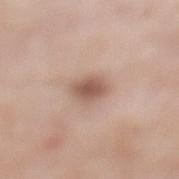workup: catalogued during a skin exam; not biopsied | anatomic site: the left lower leg | lesion size: ≈3.5 mm | lighting: white-light | image-analysis metrics: an average lesion color of about L≈56 a*≈19 b*≈26 (CIELAB), roughly 12 lightness units darker than nearby skin, and a normalized lesion–skin contrast near 8 | patient: female, in their mid- to late 50s | image source: total-body-photography crop, ~15 mm field of view.